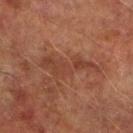Impression: The lesion was photographed on a routine skin check and not biopsied; there is no pathology result. Image and clinical context: The patient is a male aged around 75. A 15 mm close-up tile from a total-body photography series done for melanoma screening. Located on the right lower leg. Automated tile analysis of the lesion measured a mean CIELAB color near L≈33 a*≈20 b*≈25, roughly 6 lightness units darker than nearby skin, and a normalized lesion–skin contrast near 5.5. The analysis additionally found a within-lesion color-variation index near 2/10 and a peripheral color-asymmetry measure near 0.5. Approximately 7 mm at its widest.